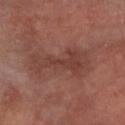Impression: Part of a total-body skin-imaging series; this lesion was reviewed on a skin check and was not flagged for biopsy. Acquisition and patient details: A roughly 15 mm field-of-view crop from a total-body skin photograph. Captured under cross-polarized illumination. The patient is a female approximately 65 years of age. The lesion is on the left arm. The recorded lesion diameter is about 7.5 mm.